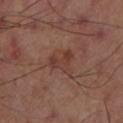Assessment: Captured during whole-body skin photography for melanoma surveillance; the lesion was not biopsied. Image and clinical context: Automated tile analysis of the lesion measured a lesion area of about 5.5 mm², a shape eccentricity near 0.75, and a symmetry-axis asymmetry near 0.5. And it measured a color-variation rating of about 5/10. The analysis additionally found a nevus-likeness score of about 5/100 and lesion-presence confidence of about 100/100. A 15 mm crop from a total-body photograph taken for skin-cancer surveillance. This is a cross-polarized tile. Located on the left thigh. Longest diameter approximately 3.5 mm.